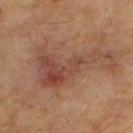• notes · total-body-photography surveillance lesion; no biopsy
• tile lighting · cross-polarized illumination
• patient · male, about 55 years old
• imaging modality · ~15 mm crop, total-body skin-cancer survey
• size · about 9 mm
• location · the upper back